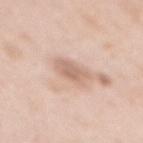workup = total-body-photography surveillance lesion; no biopsy
site = the mid back
image source = ~15 mm tile from a whole-body skin photo
lighting = white-light
subject = female, approximately 50 years of age
diameter = ≈3.5 mm
TBP lesion metrics = an area of roughly 5.5 mm² and an eccentricity of roughly 0.8; an average lesion color of about L≈65 a*≈19 b*≈28 (CIELAB) and a normalized border contrast of about 6; a border-irregularity index near 2/10, a within-lesion color-variation index near 2.5/10, and radial color variation of about 1; a detector confidence of about 100 out of 100 that the crop contains a lesion